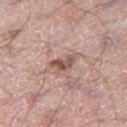The lesion was photographed on a routine skin check and not biopsied; there is no pathology result. Imaged with white-light lighting. A male patient, approximately 55 years of age. The total-body-photography lesion software estimated a footprint of about 4 mm², an eccentricity of roughly 0.85, and a shape-asymmetry score of about 0.5 (0 = symmetric). It also reported a border-irregularity index near 5/10, a color-variation rating of about 1.5/10, and peripheral color asymmetry of about 0.5. Approximately 3 mm at its widest. The lesion is located on the leg. Cropped from a whole-body photographic skin survey; the tile spans about 15 mm.